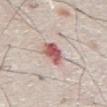A male patient, about 80 years old.
A 15 mm close-up tile from a total-body photography series done for melanoma screening.
The lesion is located on the front of the torso.
An algorithmic analysis of the crop reported border irregularity of about 2 on a 0–10 scale and internal color variation of about 6.5 on a 0–10 scale.